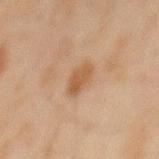Impression: Imaged during a routine full-body skin examination; the lesion was not biopsied and no histopathology is available. Clinical summary: Longest diameter approximately 3 mm. The lesion is on the mid back. The tile uses cross-polarized illumination. The subject is a male about 45 years old. A 15 mm close-up extracted from a 3D total-body photography capture.